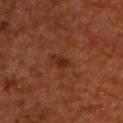subject: male, aged 53–57
lighting: cross-polarized
image-analysis metrics: an average lesion color of about L≈26 a*≈24 b*≈28 (CIELAB), roughly 6 lightness units darker than nearby skin, and a normalized border contrast of about 6.5; a within-lesion color-variation index near 1/10 and peripheral color asymmetry of about 0.5; an automated nevus-likeness rating near 15 out of 100 and a detector confidence of about 100 out of 100 that the crop contains a lesion
lesion size: ~2.5 mm (longest diameter)
site: the upper back
image source: ~15 mm crop, total-body skin-cancer survey